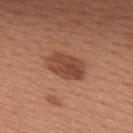Q: Is there a histopathology result?
A: no biopsy performed (imaged during a skin exam)
Q: What is the anatomic site?
A: the upper back
Q: What did automated image analysis measure?
A: an area of roughly 11 mm², a shape eccentricity near 0.8, and two-axis asymmetry of about 0.2; border irregularity of about 2 on a 0–10 scale, a within-lesion color-variation index near 3.5/10, and peripheral color asymmetry of about 1.5
Q: How was the tile lit?
A: white-light illumination
Q: What kind of image is this?
A: total-body-photography crop, ~15 mm field of view
Q: Who is the patient?
A: female, in their mid-40s
Q: How large is the lesion?
A: ~5 mm (longest diameter)